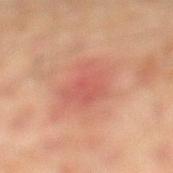Recorded during total-body skin imaging; not selected for excision or biopsy.
A roughly 15 mm field-of-view crop from a total-body skin photograph.
A male subject in their mid- to late 40s.
The recorded lesion diameter is about 5 mm.
Automated tile analysis of the lesion measured a shape eccentricity near 0.25 and a symmetry-axis asymmetry near 0.25. The analysis additionally found a normalized border contrast of about 5. The software also gave a nevus-likeness score of about 0/100.
Located on the left lower leg.